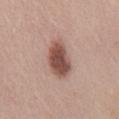The lesion was photographed on a routine skin check and not biopsied; there is no pathology result. On the mid back. The patient is a female aged 48 to 52. The lesion-visualizer software estimated a footprint of about 11 mm², an eccentricity of roughly 0.85, and a symmetry-axis asymmetry near 0.2. It also reported a mean CIELAB color near L≈50 a*≈21 b*≈25, roughly 16 lightness units darker than nearby skin, and a normalized border contrast of about 11. And it measured peripheral color asymmetry of about 1. And it measured an automated nevus-likeness rating near 95 out of 100 and a detector confidence of about 100 out of 100 that the crop contains a lesion. About 5 mm across. A close-up tile cropped from a whole-body skin photograph, about 15 mm across. This is a white-light tile.